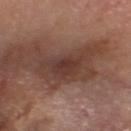biopsy status: catalogued during a skin exam; not biopsied.